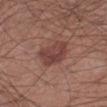No biopsy was performed on this lesion — it was imaged during a full skin examination and was not determined to be concerning. Automated image analysis of the tile measured a classifier nevus-likeness of about 70/100. The tile uses white-light illumination. The subject is a male aged approximately 45. This image is a 15 mm lesion crop taken from a total-body photograph. The recorded lesion diameter is about 4 mm. On the right lower leg.